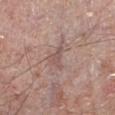Q: Is there a histopathology result?
A: imaged on a skin check; not biopsied
Q: What are the patient's age and sex?
A: male, in their mid-60s
Q: Illumination type?
A: white-light
Q: What is the anatomic site?
A: the left lower leg
Q: What is the imaging modality?
A: total-body-photography crop, ~15 mm field of view
Q: Lesion size?
A: ~2.5 mm (longest diameter)
Q: What did automated image analysis measure?
A: a shape eccentricity near 0.75 and two-axis asymmetry of about 0.55; a border-irregularity index near 5.5/10, a color-variation rating of about 1.5/10, and peripheral color asymmetry of about 0.5; an automated nevus-likeness rating near 0 out of 100 and lesion-presence confidence of about 65/100